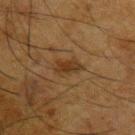follow-up = catalogued during a skin exam; not biopsied
tile lighting = cross-polarized
site = the left upper arm
patient = male, about 65 years old
imaging modality = total-body-photography crop, ~15 mm field of view
lesion size = about 3.5 mm
automated lesion analysis = internal color variation of about 2.5 on a 0–10 scale and radial color variation of about 1